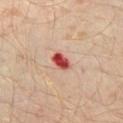follow-up — no biopsy performed (imaged during a skin exam)
image source — total-body-photography crop, ~15 mm field of view
patient — male, aged 28 to 32
size — ≈2.5 mm
illumination — cross-polarized
body site — the left thigh